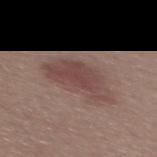workup = no biopsy performed (imaged during a skin exam)
image-analysis metrics = an average lesion color of about L≈45 a*≈18 b*≈20 (CIELAB); an automated nevus-likeness rating near 65 out of 100
illumination = white-light illumination
image source = total-body-photography crop, ~15 mm field of view
patient = female, about 25 years old
site = the upper back